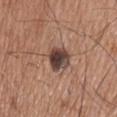| feature | finding |
|---|---|
| notes | imaged on a skin check; not biopsied |
| image-analysis metrics | a border-irregularity index near 2.5/10, a within-lesion color-variation index near 7.5/10, and peripheral color asymmetry of about 2 |
| image source | ~15 mm crop, total-body skin-cancer survey |
| location | the head or neck |
| patient | male, in their mid- to late 70s |
| lesion size | ≈3.5 mm |